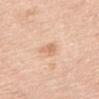notes: total-body-photography surveillance lesion; no biopsy | size: ≈3 mm | subject: male, roughly 80 years of age | acquisition: ~15 mm tile from a whole-body skin photo | body site: the mid back.